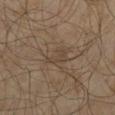No biopsy was performed on this lesion — it was imaged during a full skin examination and was not determined to be concerning. About 3 mm across. The tile uses cross-polarized illumination. Cropped from a total-body skin-imaging series; the visible field is about 15 mm. The subject is a male aged 58 to 62. The lesion is on the left thigh. The total-body-photography lesion software estimated a lesion–skin lightness drop of about 5 and a lesion-to-skin contrast of about 5 (normalized; higher = more distinct). The analysis additionally found a border-irregularity rating of about 6/10 and a within-lesion color-variation index near 0.5/10. And it measured a nevus-likeness score of about 0/100 and a lesion-detection confidence of about 80/100.